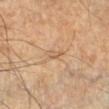  biopsy_status: not biopsied; imaged during a skin examination
  image:
    source: total-body photography crop
    field_of_view_mm: 15
  site: leg
  lighting: cross-polarized
  patient:
    sex: male
    age_approx: 55